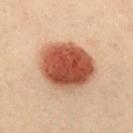workup: catalogued during a skin exam; not biopsied | subject: female, aged 28 to 32 | automated lesion analysis: a footprint of about 29 mm² and a shape-asymmetry score of about 0.1 (0 = symmetric); a border-irregularity index near 1/10, a color-variation rating of about 6/10, and a peripheral color-asymmetry measure near 1.5; a classifier nevus-likeness of about 100/100 | lesion size: ~7 mm (longest diameter) | image source: 15 mm crop, total-body photography | illumination: cross-polarized | body site: the mid back.